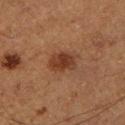Clinical impression: This lesion was catalogued during total-body skin photography and was not selected for biopsy. Image and clinical context: A close-up tile cropped from a whole-body skin photograph, about 15 mm across. The recorded lesion diameter is about 3.5 mm. A male patient, aged 73 to 77. Located on the left lower leg. The lesion-visualizer software estimated an outline eccentricity of about 0.7 (0 = round, 1 = elongated) and a shape-asymmetry score of about 0.2 (0 = symmetric). And it measured a border-irregularity rating of about 2/10, internal color variation of about 3 on a 0–10 scale, and radial color variation of about 1. It also reported a classifier nevus-likeness of about 95/100 and a lesion-detection confidence of about 100/100.